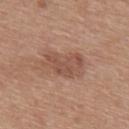Captured during whole-body skin photography for melanoma surveillance; the lesion was not biopsied.
The patient is a male aged around 30.
Captured under white-light illumination.
From the upper back.
The lesion's longest dimension is about 5 mm.
Cropped from a whole-body photographic skin survey; the tile spans about 15 mm.